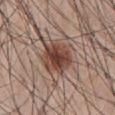Clinical impression: Captured during whole-body skin photography for melanoma surveillance; the lesion was not biopsied. Image and clinical context: Cropped from a total-body skin-imaging series; the visible field is about 15 mm. A male patient aged around 45. The tile uses white-light illumination. About 4.5 mm across. The lesion is located on the abdomen.